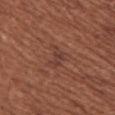Findings:
- follow-up: imaged on a skin check; not biopsied
- patient: female, roughly 65 years of age
- acquisition: 15 mm crop, total-body photography
- body site: the upper back